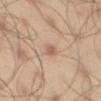follow-up: no biopsy performed (imaged during a skin exam)
location: the left thigh
automated metrics: a lesion color around L≈60 a*≈17 b*≈28 in CIELAB, about 7 CIELAB-L* units darker than the surrounding skin, and a lesion-to-skin contrast of about 4.5 (normalized; higher = more distinct); a nevus-likeness score of about 5/100 and a lesion-detection confidence of about 100/100
acquisition: ~15 mm tile from a whole-body skin photo
subject: male, in their mid-40s
illumination: cross-polarized illumination